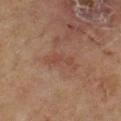{"biopsy_status": "not biopsied; imaged during a skin examination", "site": "right lower leg", "image": {"source": "total-body photography crop", "field_of_view_mm": 15}, "lighting": "cross-polarized", "lesion_size": {"long_diameter_mm_approx": 4.0}, "patient": {"sex": "female", "age_approx": 70}}